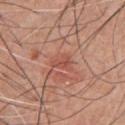Assessment:
The lesion was photographed on a routine skin check and not biopsied; there is no pathology result.
Clinical summary:
This is a white-light tile. Measured at roughly 2.5 mm in maximum diameter. Cropped from a total-body skin-imaging series; the visible field is about 15 mm. A male subject, aged 58–62. The lesion is located on the front of the torso.